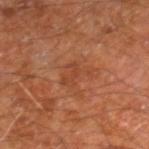biopsy_status: not biopsied; imaged during a skin examination